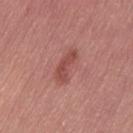The lesion-visualizer software estimated a footprint of about 6.5 mm², an outline eccentricity of about 0.9 (0 = round, 1 = elongated), and a shape-asymmetry score of about 0.35 (0 = symmetric). The analysis additionally found a lesion color around L≈49 a*≈27 b*≈25 in CIELAB and a lesion-to-skin contrast of about 6.5 (normalized; higher = more distinct).
The lesion is on the left thigh.
Measured at roughly 4.5 mm in maximum diameter.
The tile uses white-light illumination.
A female subject roughly 55 years of age.
Cropped from a whole-body photographic skin survey; the tile spans about 15 mm.